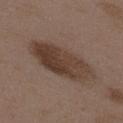Part of a total-body skin-imaging series; this lesion was reviewed on a skin check and was not flagged for biopsy. The tile uses white-light illumination. The recorded lesion diameter is about 8 mm. Cropped from a whole-body photographic skin survey; the tile spans about 15 mm. Located on the upper back. A female patient, roughly 35 years of age.